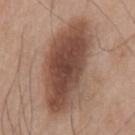Q: Is there a histopathology result?
A: total-body-photography surveillance lesion; no biopsy
Q: Lesion location?
A: the mid back
Q: Illumination type?
A: white-light illumination
Q: What is the imaging modality?
A: total-body-photography crop, ~15 mm field of view
Q: What are the patient's age and sex?
A: male, in their mid- to late 70s
Q: How large is the lesion?
A: ~11 mm (longest diameter)